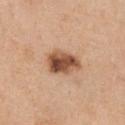Recorded during total-body skin imaging; not selected for excision or biopsy.